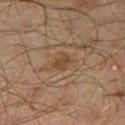Q: Was a biopsy performed?
A: total-body-photography surveillance lesion; no biopsy
Q: Patient demographics?
A: male, aged 48–52
Q: Where on the body is the lesion?
A: the right thigh
Q: What kind of image is this?
A: ~15 mm crop, total-body skin-cancer survey